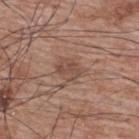follow-up: catalogued during a skin exam; not biopsied
image source: 15 mm crop, total-body photography
site: the upper back
TBP lesion metrics: a footprint of about 4.5 mm², a shape eccentricity near 0.7, and a symmetry-axis asymmetry near 0.3; an average lesion color of about L≈48 a*≈19 b*≈26 (CIELAB), a lesion–skin lightness drop of about 8, and a lesion-to-skin contrast of about 6 (normalized; higher = more distinct)
patient: male, aged 68 to 72
lesion size: ~3 mm (longest diameter)